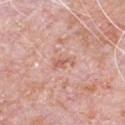Q: Is there a histopathology result?
A: no biopsy performed (imaged during a skin exam)
Q: How was this image acquired?
A: 15 mm crop, total-body photography
Q: What is the lesion's diameter?
A: ~2.5 mm (longest diameter)
Q: Where on the body is the lesion?
A: the chest
Q: What are the patient's age and sex?
A: male, aged 78–82